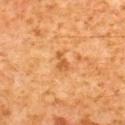Captured during whole-body skin photography for melanoma surveillance; the lesion was not biopsied. A male subject, aged 58 to 62. Measured at roughly 2.5 mm in maximum diameter. On the upper back. The lesion-visualizer software estimated an area of roughly 3 mm² and a symmetry-axis asymmetry near 0.35. It also reported internal color variation of about 1.5 on a 0–10 scale and peripheral color asymmetry of about 0.5. A 15 mm close-up extracted from a 3D total-body photography capture.